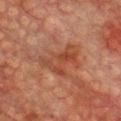The lesion was tiled from a total-body skin photograph and was not biopsied. The lesion is located on the chest. Longest diameter approximately 5 mm. The subject is a male in their mid- to late 70s. A 15 mm crop from a total-body photograph taken for skin-cancer surveillance.